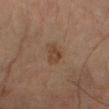| field | value |
|---|---|
| workup | imaged on a skin check; not biopsied |
| body site | the arm |
| lighting | cross-polarized |
| patient | male, aged 68–72 |
| diameter | about 2.5 mm |
| TBP lesion metrics | a footprint of about 4 mm², an eccentricity of roughly 0.75, and a symmetry-axis asymmetry near 0.4; a border-irregularity rating of about 3.5/10, a within-lesion color-variation index near 2.5/10, and a peripheral color-asymmetry measure near 1 |
| image | ~15 mm crop, total-body skin-cancer survey |